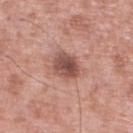This lesion was catalogued during total-body skin photography and was not selected for biopsy.
The lesion is on the right lower leg.
Measured at roughly 3.5 mm in maximum diameter.
An algorithmic analysis of the crop reported a lesion color around L≈50 a*≈23 b*≈24 in CIELAB and a normalized lesion–skin contrast near 9.5. The analysis additionally found a border-irregularity index near 1.5/10 and a within-lesion color-variation index near 4.5/10.
A male patient, about 55 years old.
Imaged with white-light lighting.
A roughly 15 mm field-of-view crop from a total-body skin photograph.